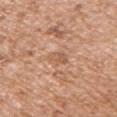This lesion was catalogued during total-body skin photography and was not selected for biopsy. Located on the right upper arm. Approximately 2.5 mm at its widest. Imaged with white-light lighting. A close-up tile cropped from a whole-body skin photograph, about 15 mm across. A male patient about 75 years old. The lesion-visualizer software estimated a lesion area of about 3.5 mm² and two-axis asymmetry of about 0.3. The analysis additionally found a lesion color around L≈57 a*≈21 b*≈33 in CIELAB and a normalized lesion–skin contrast near 5.5. And it measured a border-irregularity rating of about 3/10, a within-lesion color-variation index near 2.5/10, and a peripheral color-asymmetry measure near 1. The analysis additionally found a lesion-detection confidence of about 95/100.